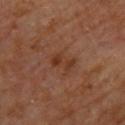The lesion was photographed on a routine skin check and not biopsied; there is no pathology result. Approximately 3 mm at its widest. A close-up tile cropped from a whole-body skin photograph, about 15 mm across. A male subject, aged around 60. On the upper back.